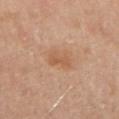A female patient aged 48–52. The lesion is located on the left upper arm. A close-up tile cropped from a whole-body skin photograph, about 15 mm across.